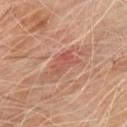<record>
<biopsy_status>not biopsied; imaged during a skin examination</biopsy_status>
<patient>
  <sex>male</sex>
  <age_approx>70</age_approx>
</patient>
<site>chest</site>
<image>
  <source>total-body photography crop</source>
  <field_of_view_mm>15</field_of_view_mm>
</image>
</record>